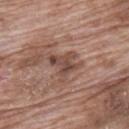No biopsy was performed on this lesion — it was imaged during a full skin examination and was not determined to be concerning.
The lesion is located on the upper back.
Cropped from a total-body skin-imaging series; the visible field is about 15 mm.
This is a white-light tile.
The subject is a male about 70 years old.
Measured at roughly 3.5 mm in maximum diameter.